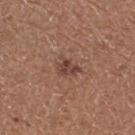Captured during whole-body skin photography for melanoma surveillance; the lesion was not biopsied. The lesion is located on the right thigh. A region of skin cropped from a whole-body photographic capture, roughly 15 mm wide. Longest diameter approximately 3 mm. Captured under white-light illumination. The patient is a female aged 33–37.